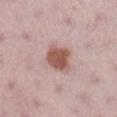imaging modality: 15 mm crop, total-body photography
subject: female, aged 28 to 32
site: the leg